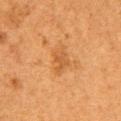Impression: The lesion was tiled from a total-body skin photograph and was not biopsied. Background: Longest diameter approximately 3 mm. A 15 mm crop from a total-body photograph taken for skin-cancer surveillance. The lesion is on the chest. Captured under cross-polarized illumination. A female patient, aged around 50. An algorithmic analysis of the crop reported two-axis asymmetry of about 0.3. And it measured a nevus-likeness score of about 20/100 and a detector confidence of about 100 out of 100 that the crop contains a lesion.